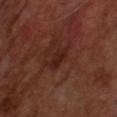Impression: Part of a total-body skin-imaging series; this lesion was reviewed on a skin check and was not flagged for biopsy. Context: This is a cross-polarized tile. A male subject approximately 65 years of age. A close-up tile cropped from a whole-body skin photograph, about 15 mm across. About 3 mm across.